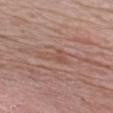Assessment:
Captured during whole-body skin photography for melanoma surveillance; the lesion was not biopsied.
Context:
A male subject aged approximately 65. Approximately 4 mm at its widest. Automated image analysis of the tile measured a lesion area of about 4.5 mm², an eccentricity of roughly 0.9, and a shape-asymmetry score of about 0.65 (0 = symmetric). The analysis additionally found a classifier nevus-likeness of about 0/100. Cropped from a whole-body photographic skin survey; the tile spans about 15 mm. From the chest. Captured under white-light illumination.